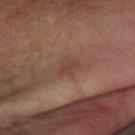Notes:
• notes · imaged on a skin check; not biopsied
• illumination · cross-polarized illumination
• subject · male, aged 58–62
• location · the arm
• lesion diameter · ~2.5 mm (longest diameter)
• image source · total-body-photography crop, ~15 mm field of view